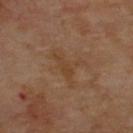Captured during whole-body skin photography for melanoma surveillance; the lesion was not biopsied.
The lesion-visualizer software estimated border irregularity of about 8 on a 0–10 scale, internal color variation of about 1 on a 0–10 scale, and radial color variation of about 0.5.
Captured under cross-polarized illumination.
Located on the upper back.
A lesion tile, about 15 mm wide, cut from a 3D total-body photograph.
The lesion's longest dimension is about 5 mm.
A male patient approximately 70 years of age.